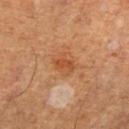biopsy_status: not biopsied; imaged during a skin examination
image:
  source: total-body photography crop
  field_of_view_mm: 15
site: left lower leg
lesion_size:
  long_diameter_mm_approx: 3.0
lighting: cross-polarized
patient:
  sex: male
  age_approx: 60